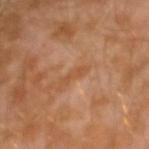Context: From the left arm. The patient is a male aged 28 to 32. A region of skin cropped from a whole-body photographic capture, roughly 15 mm wide.